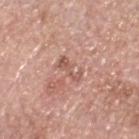Q: Was this lesion biopsied?
A: total-body-photography surveillance lesion; no biopsy
Q: What are the patient's age and sex?
A: male, roughly 60 years of age
Q: What is the imaging modality?
A: 15 mm crop, total-body photography
Q: What is the anatomic site?
A: the head or neck